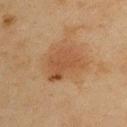Clinical impression:
Part of a total-body skin-imaging series; this lesion was reviewed on a skin check and was not flagged for biopsy.
Context:
A roughly 15 mm field-of-view crop from a total-body skin photograph. A male subject aged 43 to 47. The lesion's longest dimension is about 5 mm. The lesion is on the left upper arm. The tile uses cross-polarized illumination.